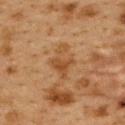Captured during whole-body skin photography for melanoma surveillance; the lesion was not biopsied. This is a cross-polarized tile. A female subject, in their 40s. The lesion is located on the upper back. About 4 mm across. A region of skin cropped from a whole-body photographic capture, roughly 15 mm wide. An algorithmic analysis of the crop reported a lesion-detection confidence of about 100/100.